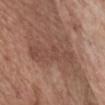workup: no biopsy performed (imaged during a skin exam)
size: about 7.5 mm
subject: male, about 55 years old
anatomic site: the chest
image: ~15 mm tile from a whole-body skin photo